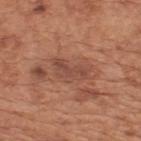Image and clinical context: About 4.5 mm across. A male patient, aged around 65. Cropped from a total-body skin-imaging series; the visible field is about 15 mm. The lesion is located on the upper back.